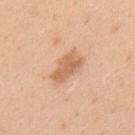Captured during whole-body skin photography for melanoma surveillance; the lesion was not biopsied. From the upper back. Longest diameter approximately 4.5 mm. A region of skin cropped from a whole-body photographic capture, roughly 15 mm wide. A male subject, in their mid- to late 30s. Captured under white-light illumination.